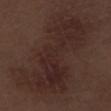{
  "biopsy_status": "not biopsied; imaged during a skin examination",
  "automated_metrics": {
    "border_irregularity_0_10": 7.0,
    "peripheral_color_asymmetry": 1.0,
    "nevus_likeness_0_100": 0,
    "lesion_detection_confidence_0_100": 100
  },
  "lighting": "white-light",
  "site": "leg",
  "lesion_size": {
    "long_diameter_mm_approx": 14.0
  },
  "patient": {
    "sex": "male",
    "age_approx": 70
  },
  "image": {
    "source": "total-body photography crop",
    "field_of_view_mm": 15
  }
}